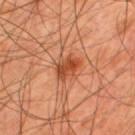Part of a total-body skin-imaging series; this lesion was reviewed on a skin check and was not flagged for biopsy. The lesion-visualizer software estimated an area of roughly 6 mm², an eccentricity of roughly 0.75, and a shape-asymmetry score of about 0.25 (0 = symmetric). The software also gave border irregularity of about 3 on a 0–10 scale, a color-variation rating of about 4.5/10, and peripheral color asymmetry of about 2. And it measured lesion-presence confidence of about 100/100. Longest diameter approximately 3 mm. A male patient in their mid- to late 40s. A lesion tile, about 15 mm wide, cut from a 3D total-body photograph. Imaged with cross-polarized lighting. From the upper back.